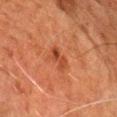Recorded during total-body skin imaging; not selected for excision or biopsy.
The lesion is located on the chest.
A male subject, about 80 years old.
About 3 mm across.
An algorithmic analysis of the crop reported a border-irregularity index near 4.5/10 and radial color variation of about 0.
The tile uses cross-polarized illumination.
A region of skin cropped from a whole-body photographic capture, roughly 15 mm wide.